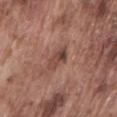Q: Was a biopsy performed?
A: imaged on a skin check; not biopsied
Q: What is the anatomic site?
A: the lower back
Q: What are the patient's age and sex?
A: male, roughly 75 years of age
Q: How was this image acquired?
A: 15 mm crop, total-body photography